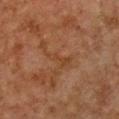workup: catalogued during a skin exam; not biopsied
image: total-body-photography crop, ~15 mm field of view
anatomic site: the chest
illumination: cross-polarized illumination
TBP lesion metrics: an average lesion color of about L≈37 a*≈20 b*≈31 (CIELAB) and a lesion-to-skin contrast of about 5 (normalized; higher = more distinct)
size: ~4 mm (longest diameter)
patient: female, roughly 60 years of age